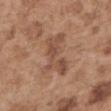<tbp_lesion>
<site>left upper arm</site>
<patient>
  <sex>male</sex>
  <age_approx>55</age_approx>
</patient>
<lighting>white-light</lighting>
<lesion_size>
  <long_diameter_mm_approx>6.0</long_diameter_mm_approx>
</lesion_size>
<image>
  <source>total-body photography crop</source>
  <field_of_view_mm>15</field_of_view_mm>
</image>
</tbp_lesion>